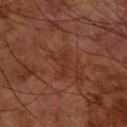workup: imaged on a skin check; not biopsied | subject: male, roughly 60 years of age | lesion diameter: ≈3 mm | image source: ~15 mm tile from a whole-body skin photo | anatomic site: the right forearm | TBP lesion metrics: a footprint of about 3.5 mm², an outline eccentricity of about 0.9 (0 = round, 1 = elongated), and a symmetry-axis asymmetry near 0.5; a border-irregularity rating of about 5/10, a within-lesion color-variation index near 1/10, and a peripheral color-asymmetry measure near 0.5.